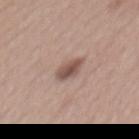Context: On the mid back. About 3.5 mm across. A 15 mm crop from a total-body photograph taken for skin-cancer surveillance. A female patient aged around 55. The tile uses white-light illumination.